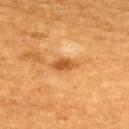workup: imaged on a skin check; not biopsied
body site: the upper back
subject: male, aged 58 to 62
image source: ~15 mm crop, total-body skin-cancer survey
size: about 3 mm
tile lighting: cross-polarized
image-analysis metrics: a lesion area of about 4 mm², a shape eccentricity near 0.85, and two-axis asymmetry of about 0.3; a mean CIELAB color near L≈51 a*≈26 b*≈43, a lesion–skin lightness drop of about 12, and a lesion-to-skin contrast of about 8 (normalized; higher = more distinct); a classifier nevus-likeness of about 50/100 and a lesion-detection confidence of about 100/100